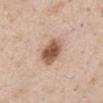<tbp_lesion>
<biopsy_status>not biopsied; imaged during a skin examination</biopsy_status>
<lesion_size>
  <long_diameter_mm_approx>4.5</long_diameter_mm_approx>
</lesion_size>
<patient>
  <sex>male</sex>
  <age_approx>60</age_approx>
</patient>
<site>abdomen</site>
<lighting>white-light</lighting>
<image>
  <source>total-body photography crop</source>
  <field_of_view_mm>15</field_of_view_mm>
</image>
</tbp_lesion>